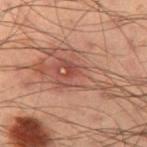workup: total-body-photography surveillance lesion; no biopsy
patient: male, approximately 55 years of age
image: ~15 mm crop, total-body skin-cancer survey
diameter: about 9.5 mm
body site: the left thigh
lighting: cross-polarized illumination